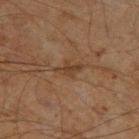Case summary:
* workup · catalogued during a skin exam; not biopsied
* image source · 15 mm crop, total-body photography
* size · ~3 mm (longest diameter)
* subject · male, roughly 60 years of age
* lighting · cross-polarized
* location · the left thigh